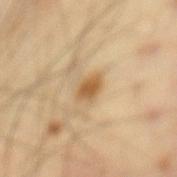Q: Was this lesion biopsied?
A: no biopsy performed (imaged during a skin exam)